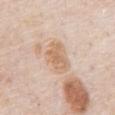{"biopsy_status": "not biopsied; imaged during a skin examination", "lighting": "white-light", "image": {"source": "total-body photography crop", "field_of_view_mm": 15}, "site": "front of the torso", "patient": {"sex": "male", "age_approx": 80}, "lesion_size": {"long_diameter_mm_approx": 4.5}, "automated_metrics": {"cielab_L": 67, "cielab_a": 17, "cielab_b": 32, "vs_skin_darker_L": 9.0, "vs_skin_contrast_norm": 7.0, "border_irregularity_0_10": 3.0, "color_variation_0_10": 3.0, "peripheral_color_asymmetry": 1.0, "lesion_detection_confidence_0_100": 100}}